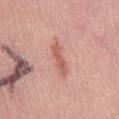acquisition: ~15 mm crop, total-body skin-cancer survey | site: the abdomen | subject: male, aged 28–32 | pathology: a compound melanocytic nevus.